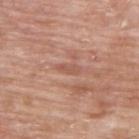Q: What kind of image is this?
A: 15 mm crop, total-body photography
Q: Where on the body is the lesion?
A: the upper back
Q: Patient demographics?
A: male, aged around 65